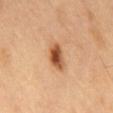Findings:
* biopsy status — no biopsy performed (imaged during a skin exam)
* acquisition — ~15 mm crop, total-body skin-cancer survey
* body site — the back
* subject — male, in their 60s
* diameter — ~3.5 mm (longest diameter)
* tile lighting — cross-polarized
* TBP lesion metrics — a lesion area of about 6.5 mm², an outline eccentricity of about 0.75 (0 = round, 1 = elongated), and a symmetry-axis asymmetry near 0.2; roughly 15 lightness units darker than nearby skin and a lesion-to-skin contrast of about 10.5 (normalized; higher = more distinct); a border-irregularity index near 2/10, internal color variation of about 7 on a 0–10 scale, and a peripheral color-asymmetry measure near 2